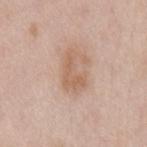Q: Was a biopsy performed?
A: no biopsy performed (imaged during a skin exam)
Q: Illumination type?
A: white-light
Q: How was this image acquired?
A: total-body-photography crop, ~15 mm field of view
Q: What are the patient's age and sex?
A: male, aged around 55
Q: How large is the lesion?
A: ~4.5 mm (longest diameter)
Q: What is the anatomic site?
A: the chest